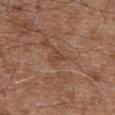No biopsy was performed on this lesion — it was imaged during a full skin examination and was not determined to be concerning. A 15 mm close-up extracted from a 3D total-body photography capture. From the abdomen. A male subject approximately 75 years of age.